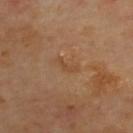Notes:
- workup: total-body-photography surveillance lesion; no biopsy
- image source: total-body-photography crop, ~15 mm field of view
- lesion size: about 2.5 mm
- location: the upper back
- tile lighting: cross-polarized illumination